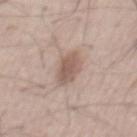{"biopsy_status": "not biopsied; imaged during a skin examination", "image": {"source": "total-body photography crop", "field_of_view_mm": 15}, "patient": {"sex": "male", "age_approx": 55}, "automated_metrics": {"vs_skin_darker_L": 11.0, "vs_skin_contrast_norm": 7.5, "nevus_likeness_0_100": 85}}